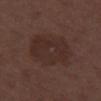This lesion was catalogued during total-body skin photography and was not selected for biopsy.
This is a white-light tile.
The lesion is located on the right thigh.
About 6.5 mm across.
A 15 mm crop from a total-body photograph taken for skin-cancer surveillance.
A female subject approximately 50 years of age.